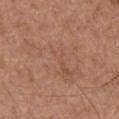workup=total-body-photography surveillance lesion; no biopsy
subject=male, aged 63–67
body site=the front of the torso
imaging modality=~15 mm crop, total-body skin-cancer survey